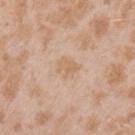Imaged during a routine full-body skin examination; the lesion was not biopsied and no histopathology is available.
A female subject aged approximately 25.
About 2.5 mm across.
The lesion is on the left upper arm.
An algorithmic analysis of the crop reported an area of roughly 5 mm², a shape eccentricity near 0.55, and a shape-asymmetry score of about 0.25 (0 = symmetric).
A region of skin cropped from a whole-body photographic capture, roughly 15 mm wide.
Captured under white-light illumination.